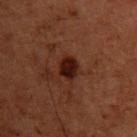  biopsy_status: not biopsied; imaged during a skin examination
  site: upper back
  lesion_size:
    long_diameter_mm_approx: 2.5
  patient:
    sex: male
    age_approx: 60
  automated_metrics:
    eccentricity: 0.6
    vs_skin_contrast_norm: 12.5
    border_irregularity_0_10: 2.0
    color_variation_0_10: 2.0
    peripheral_color_asymmetry: 0.5
    nevus_likeness_0_100: 85
  image:
    source: total-body photography crop
    field_of_view_mm: 15
  lighting: cross-polarized